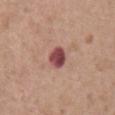  biopsy_status: not biopsied; imaged during a skin examination
  lesion_size:
    long_diameter_mm_approx: 3.0
  lighting: white-light
  image:
    source: total-body photography crop
    field_of_view_mm: 15
  site: abdomen
  patient:
    sex: female
    age_approx: 75